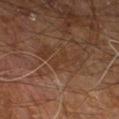The lesion was photographed on a routine skin check and not biopsied; there is no pathology result.
The subject is a male about 60 years old.
Captured under cross-polarized illumination.
Automated tile analysis of the lesion measured a mean CIELAB color near L≈34 a*≈18 b*≈29. And it measured a lesion-detection confidence of about 55/100.
On the right leg.
A close-up tile cropped from a whole-body skin photograph, about 15 mm across.
The recorded lesion diameter is about 2.5 mm.